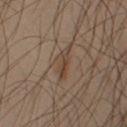biopsy status=total-body-photography surveillance lesion; no biopsy | tile lighting=cross-polarized | image=total-body-photography crop, ~15 mm field of view | location=the right upper arm | patient=male, in their 40s.